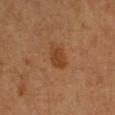notes: no biopsy performed (imaged during a skin exam) | site: the left forearm | lesion diameter: about 3 mm | image: total-body-photography crop, ~15 mm field of view | subject: female, aged 38–42 | automated metrics: an outline eccentricity of about 0.6 (0 = round, 1 = elongated) and a shape-asymmetry score of about 0.2 (0 = symmetric); a lesion color around L≈35 a*≈20 b*≈31 in CIELAB, a lesion–skin lightness drop of about 7, and a normalized border contrast of about 7; a border-irregularity rating of about 2/10; lesion-presence confidence of about 100/100.